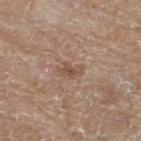- biopsy status: total-body-photography surveillance lesion; no biopsy
- size: ~3 mm (longest diameter)
- patient: female, roughly 75 years of age
- image: ~15 mm tile from a whole-body skin photo
- lighting: white-light illumination
- image-analysis metrics: a classifier nevus-likeness of about 0/100
- location: the leg